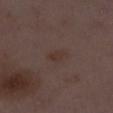This lesion was catalogued during total-body skin photography and was not selected for biopsy.
The subject is a female about 30 years old.
A roughly 15 mm field-of-view crop from a total-body skin photograph.
Measured at roughly 2.5 mm in maximum diameter.
The lesion is on the right lower leg.
The tile uses white-light illumination.